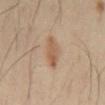Q: Was this lesion biopsied?
A: total-body-photography surveillance lesion; no biopsy
Q: How large is the lesion?
A: about 4.5 mm
Q: What is the anatomic site?
A: the chest
Q: What lighting was used for the tile?
A: cross-polarized illumination
Q: Patient demographics?
A: male, aged 38 to 42
Q: What kind of image is this?
A: ~15 mm crop, total-body skin-cancer survey
Q: Automated lesion metrics?
A: two-axis asymmetry of about 0.35; an average lesion color of about L≈54 a*≈18 b*≈32 (CIELAB) and about 9 CIELAB-L* units darker than the surrounding skin